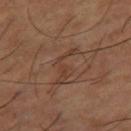Recorded during total-body skin imaging; not selected for excision or biopsy. The patient is a male approximately 65 years of age. A close-up tile cropped from a whole-body skin photograph, about 15 mm across. Located on the leg. Automated tile analysis of the lesion measured an average lesion color of about L≈38 a*≈18 b*≈28 (CIELAB), about 6 CIELAB-L* units darker than the surrounding skin, and a normalized border contrast of about 5.5. It also reported border irregularity of about 10 on a 0–10 scale and a peripheral color-asymmetry measure near 0. It also reported a nevus-likeness score of about 0/100 and lesion-presence confidence of about 80/100. The tile uses cross-polarized illumination. Longest diameter approximately 5 mm.